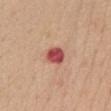The lesion was photographed on a routine skin check and not biopsied; there is no pathology result. The total-body-photography lesion software estimated an average lesion color of about L≈50 a*≈35 b*≈27 (CIELAB), about 17 CIELAB-L* units darker than the surrounding skin, and a normalized lesion–skin contrast near 11.5. The software also gave a border-irregularity rating of about 1.5/10, a color-variation rating of about 3.5/10, and a peripheral color-asymmetry measure near 1. Located on the front of the torso. A female patient, aged 58–62. About 2.5 mm across. This image is a 15 mm lesion crop taken from a total-body photograph. Imaged with white-light lighting.